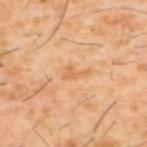Case summary:
– biopsy status: catalogued during a skin exam; not biopsied
– diameter: about 2.5 mm
– illumination: cross-polarized illumination
– acquisition: ~15 mm crop, total-body skin-cancer survey
– location: the back
– subject: male, approximately 60 years of age